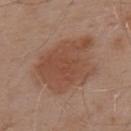Imaged during a routine full-body skin examination; the lesion was not biopsied and no histopathology is available.